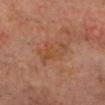This lesion was catalogued during total-body skin photography and was not selected for biopsy. A 15 mm close-up extracted from a 3D total-body photography capture. Automated image analysis of the tile measured a lesion area of about 8 mm², an outline eccentricity of about 0.9 (0 = round, 1 = elongated), and two-axis asymmetry of about 0.4. On the head or neck. Measured at roughly 5 mm in maximum diameter. The subject is a male about 60 years old.